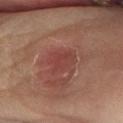Part of a total-body skin-imaging series; this lesion was reviewed on a skin check and was not flagged for biopsy. The total-body-photography lesion software estimated a mean CIELAB color near L≈41 a*≈26 b*≈24, roughly 6 lightness units darker than nearby skin, and a normalized lesion–skin contrast near 5.5. And it measured internal color variation of about 2.5 on a 0–10 scale and a peripheral color-asymmetry measure near 1. The lesion is located on the left lower leg. Cropped from a whole-body photographic skin survey; the tile spans about 15 mm. About 3.5 mm across. This is a cross-polarized tile. The patient is a female aged 48–52.